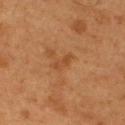The lesion was photographed on a routine skin check and not biopsied; there is no pathology result. Automated image analysis of the tile measured border irregularity of about 3.5 on a 0–10 scale, a within-lesion color-variation index near 0/10, and a peripheral color-asymmetry measure near 0. The software also gave a nevus-likeness score of about 0/100 and a lesion-detection confidence of about 100/100. On the upper back. A male subject, approximately 55 years of age. A close-up tile cropped from a whole-body skin photograph, about 15 mm across.